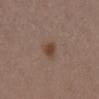This lesion was catalogued during total-body skin photography and was not selected for biopsy. A female subject, aged around 40. A 15 mm close-up extracted from a 3D total-body photography capture. From the right lower leg. Captured under white-light illumination. The lesion's longest dimension is about 2.5 mm.